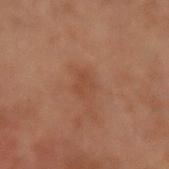• follow-up · imaged on a skin check; not biopsied
• tile lighting · cross-polarized illumination
• location · the left forearm
• image · ~15 mm crop, total-body skin-cancer survey
• patient · male, aged 58–62
• automated lesion analysis · a footprint of about 4 mm², an eccentricity of roughly 0.7, and two-axis asymmetry of about 0.4; roughly 5 lightness units darker than nearby skin and a normalized lesion–skin contrast near 4.5
• size · about 2.5 mm